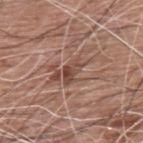Clinical impression:
Captured during whole-body skin photography for melanoma surveillance; the lesion was not biopsied.
Context:
A 15 mm close-up extracted from a 3D total-body photography capture. The lesion is located on the back. An algorithmic analysis of the crop reported a border-irregularity index near 4.5/10, a color-variation rating of about 5.5/10, and peripheral color asymmetry of about 2.5. This is a white-light tile. The recorded lesion diameter is about 3.5 mm. The patient is a male aged approximately 60.